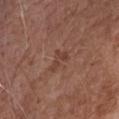biopsy status = catalogued during a skin exam; not biopsied
subject = female, aged 73–77
anatomic site = the head or neck
image = total-body-photography crop, ~15 mm field of view